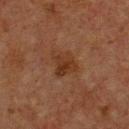Findings:
* biopsy status · imaged on a skin check; not biopsied
* acquisition · ~15 mm tile from a whole-body skin photo
* patient · male, aged 73–77
* body site · the chest